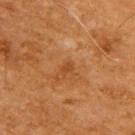Q: Is there a histopathology result?
A: catalogued during a skin exam; not biopsied
Q: Where on the body is the lesion?
A: the upper back
Q: How was this image acquired?
A: 15 mm crop, total-body photography
Q: How was the tile lit?
A: cross-polarized illumination
Q: Who is the patient?
A: male, aged 58 to 62